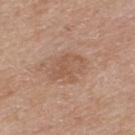biopsy status: no biopsy performed (imaged during a skin exam) | patient: male, approximately 75 years of age | illumination: white-light illumination | imaging modality: total-body-photography crop, ~15 mm field of view | anatomic site: the back.